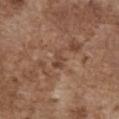Q: Is there a histopathology result?
A: no biopsy performed (imaged during a skin exam)